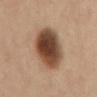This lesion was catalogued during total-body skin photography and was not selected for biopsy. Automated image analysis of the tile measured a border-irregularity rating of about 1.5/10 and peripheral color asymmetry of about 2. Longest diameter approximately 6.5 mm. Located on the mid back. A female patient roughly 45 years of age. A region of skin cropped from a whole-body photographic capture, roughly 15 mm wide.